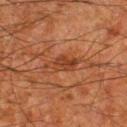Clinical impression: The lesion was tiled from a total-body skin photograph and was not biopsied. Clinical summary: Measured at roughly 4 mm in maximum diameter. Located on the leg. A close-up tile cropped from a whole-body skin photograph, about 15 mm across. Imaged with cross-polarized lighting. A male subject, aged around 80.